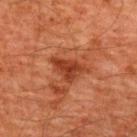No biopsy was performed on this lesion — it was imaged during a full skin examination and was not determined to be concerning. Located on the upper back. A 15 mm crop from a total-body photograph taken for skin-cancer surveillance. The tile uses cross-polarized illumination. The lesion's longest dimension is about 4.5 mm. The subject is a male approximately 60 years of age.